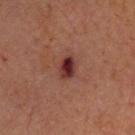workup: total-body-photography surveillance lesion; no biopsy | diameter: about 3 mm | patient: male, in their 70s | image: ~15 mm tile from a whole-body skin photo | site: the head or neck | illumination: cross-polarized illumination.